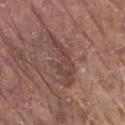biopsy status: imaged on a skin check; not biopsied
subject: male, aged approximately 80
lighting: white-light
anatomic site: the right thigh
imaging modality: ~15 mm tile from a whole-body skin photo
size: ≈7 mm